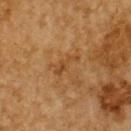Q: Is there a histopathology result?
A: catalogued during a skin exam; not biopsied
Q: What are the patient's age and sex?
A: male, aged approximately 85 or older
Q: How was the tile lit?
A: cross-polarized illumination
Q: What is the anatomic site?
A: the upper back
Q: How was this image acquired?
A: ~15 mm crop, total-body skin-cancer survey